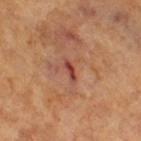Recorded during total-body skin imaging; not selected for excision or biopsy.
Located on the right thigh.
A female patient, approximately 55 years of age.
Approximately 2.5 mm at its widest.
A region of skin cropped from a whole-body photographic capture, roughly 15 mm wide.
Captured under cross-polarized illumination.